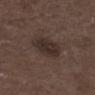Image and clinical context:
On the leg. Captured under white-light illumination. The patient is a male aged around 75. A 15 mm close-up tile from a total-body photography series done for melanoma screening. Longest diameter approximately 4 mm.